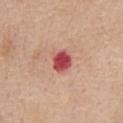Q: Was a biopsy performed?
A: imaged on a skin check; not biopsied
Q: How was this image acquired?
A: 15 mm crop, total-body photography
Q: What are the patient's age and sex?
A: female, in their mid- to late 60s
Q: What is the lesion's diameter?
A: ≈3 mm
Q: Automated lesion metrics?
A: a footprint of about 5.5 mm², an eccentricity of roughly 0.55, and a symmetry-axis asymmetry near 0.2
Q: Where on the body is the lesion?
A: the chest
Q: What lighting was used for the tile?
A: white-light illumination